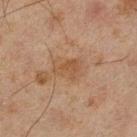notes: no biopsy performed (imaged during a skin exam)
patient: male, aged around 45
imaging modality: total-body-photography crop, ~15 mm field of view
body site: the left lower leg
lesion size: ≈3.5 mm